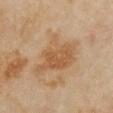workup = imaged on a skin check; not biopsied | anatomic site = the chest | diameter = ~6 mm (longest diameter) | image source = ~15 mm crop, total-body skin-cancer survey | subject = female, aged 33 to 37.